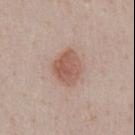biopsy_status: not biopsied; imaged during a skin examination
image:
  source: total-body photography crop
  field_of_view_mm: 15
lesion_size:
  long_diameter_mm_approx: 4.0
site: abdomen
lighting: white-light
patient:
  sex: male
  age_approx: 40